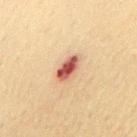Notes:
• notes: total-body-photography surveillance lesion; no biopsy
• patient: male, aged 68 to 72
• body site: the left upper arm
• lesion size: ~4 mm (longest diameter)
• image source: ~15 mm crop, total-body skin-cancer survey